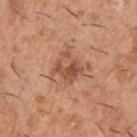{
  "biopsy_status": "not biopsied; imaged during a skin examination",
  "patient": {
    "sex": "male",
    "age_approx": 40
  },
  "automated_metrics": {
    "area_mm2_approx": 7.5,
    "eccentricity": 0.4,
    "cielab_L": 52,
    "cielab_a": 24,
    "cielab_b": 32,
    "vs_skin_darker_L": 10.0,
    "vs_skin_contrast_norm": 7.0
  },
  "lighting": "white-light",
  "site": "upper back",
  "image": {
    "source": "total-body photography crop",
    "field_of_view_mm": 15
  }
}